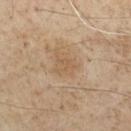{
  "biopsy_status": "not biopsied; imaged during a skin examination",
  "patient": {
    "sex": "male",
    "age_approx": 70
  },
  "site": "chest",
  "image": {
    "source": "total-body photography crop",
    "field_of_view_mm": 15
  },
  "automated_metrics": {
    "cielab_L": 55,
    "cielab_a": 15,
    "cielab_b": 32,
    "vs_skin_darker_L": 6.0,
    "vs_skin_contrast_norm": 4.5,
    "border_irregularity_0_10": 4.5,
    "color_variation_0_10": 2.0,
    "peripheral_color_asymmetry": 0.5
  },
  "lighting": "cross-polarized"
}